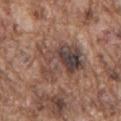{
  "biopsy_status": "not biopsied; imaged during a skin examination",
  "lighting": "white-light",
  "image": {
    "source": "total-body photography crop",
    "field_of_view_mm": 15
  },
  "site": "back",
  "lesion_size": {
    "long_diameter_mm_approx": 6.0
  },
  "patient": {
    "sex": "male",
    "age_approx": 75
  }
}